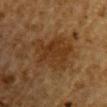Clinical impression:
No biopsy was performed on this lesion — it was imaged during a full skin examination and was not determined to be concerning.
Background:
About 5.5 mm across. On the right upper arm. Cropped from a whole-body photographic skin survey; the tile spans about 15 mm. Automated image analysis of the tile measured a mean CIELAB color near L≈30 a*≈17 b*≈30, roughly 6 lightness units darker than nearby skin, and a normalized lesion–skin contrast near 7. A male subject, roughly 85 years of age or older.